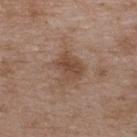site: the back; lesion diameter: about 3.5 mm; imaging modality: 15 mm crop, total-body photography; subject: male, approximately 50 years of age; tile lighting: white-light.